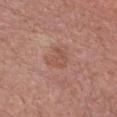Q: Was this lesion biopsied?
A: no biopsy performed (imaged during a skin exam)
Q: Lesion size?
A: ~2.5 mm (longest diameter)
Q: Illumination type?
A: white-light
Q: Automated lesion metrics?
A: a lesion area of about 5 mm², a shape eccentricity near 0.55, and a shape-asymmetry score of about 0.45 (0 = symmetric); an average lesion color of about L≈52 a*≈23 b*≈28 (CIELAB) and a normalized border contrast of about 5.5
Q: Lesion location?
A: the chest
Q: How was this image acquired?
A: ~15 mm crop, total-body skin-cancer survey
Q: What are the patient's age and sex?
A: female, aged around 60